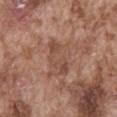The lesion was tiled from a total-body skin photograph and was not biopsied. Automated tile analysis of the lesion measured border irregularity of about 6 on a 0–10 scale and a color-variation rating of about 3/10. It also reported a nevus-likeness score of about 0/100. A male subject in their mid-70s. The lesion is on the abdomen. A roughly 15 mm field-of-view crop from a total-body skin photograph.